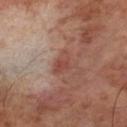{
  "biopsy_status": "not biopsied; imaged during a skin examination",
  "automated_metrics": {
    "area_mm2_approx": 4.5,
    "eccentricity": 0.8,
    "shape_asymmetry": 0.4,
    "nevus_likeness_0_100": 5,
    "lesion_detection_confidence_0_100": 100
  },
  "site": "left lower leg",
  "lighting": "cross-polarized",
  "patient": {
    "sex": "male",
    "age_approx": 70
  },
  "image": {
    "source": "total-body photography crop",
    "field_of_view_mm": 15
  },
  "lesion_size": {
    "long_diameter_mm_approx": 3.0
  }
}